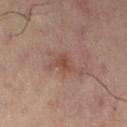Assessment:
Recorded during total-body skin imaging; not selected for excision or biopsy.
Background:
The tile uses cross-polarized illumination. A female subject aged 53–57. Approximately 3 mm at its widest. An algorithmic analysis of the crop reported a footprint of about 4.5 mm² and a shape eccentricity near 0.8. The software also gave a lesion color around L≈39 a*≈17 b*≈21 in CIELAB, roughly 6 lightness units darker than nearby skin, and a normalized lesion–skin contrast near 6. Located on the left leg. A lesion tile, about 15 mm wide, cut from a 3D total-body photograph.